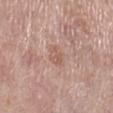Captured during whole-body skin photography for melanoma surveillance; the lesion was not biopsied. Cropped from a total-body skin-imaging series; the visible field is about 15 mm. A female patient, aged around 70. On the right lower leg.